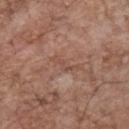follow-up: imaged on a skin check; not biopsied
tile lighting: white-light
subject: male, in their 70s
imaging modality: ~15 mm tile from a whole-body skin photo
size: ≈3 mm
anatomic site: the chest
automated lesion analysis: a mean CIELAB color near L≈49 a*≈20 b*≈27, about 6 CIELAB-L* units darker than the surrounding skin, and a normalized border contrast of about 4.5; an automated nevus-likeness rating near 0 out of 100 and lesion-presence confidence of about 85/100